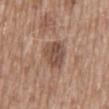The lesion was photographed on a routine skin check and not biopsied; there is no pathology result. Cropped from a total-body skin-imaging series; the visible field is about 15 mm. About 4 mm across. This is a white-light tile. The patient is a male in their 60s. From the abdomen.